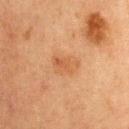Captured during whole-body skin photography for melanoma surveillance; the lesion was not biopsied.
The lesion-visualizer software estimated a lesion area of about 7 mm², a shape eccentricity near 0.75, and two-axis asymmetry of about 0.2. The software also gave a border-irregularity index near 2/10 and a color-variation rating of about 3.5/10. And it measured a classifier nevus-likeness of about 0/100 and a detector confidence of about 100 out of 100 that the crop contains a lesion.
A close-up tile cropped from a whole-body skin photograph, about 15 mm across.
Longest diameter approximately 3.5 mm.
The lesion is located on the upper back.
Captured under cross-polarized illumination.
The patient is a female aged 58 to 62.